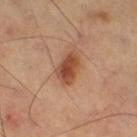No biopsy was performed on this lesion — it was imaged during a full skin examination and was not determined to be concerning.
Cropped from a whole-body photographic skin survey; the tile spans about 15 mm.
This is a cross-polarized tile.
The total-body-photography lesion software estimated a border-irregularity rating of about 2/10 and radial color variation of about 1. And it measured a classifier nevus-likeness of about 100/100 and a detector confidence of about 100 out of 100 that the crop contains a lesion.
On the right thigh.
Measured at roughly 4 mm in maximum diameter.